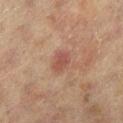Notes:
• biopsy status · catalogued during a skin exam; not biopsied
• subject · female, aged around 80
• acquisition · 15 mm crop, total-body photography
• site · the left lower leg
• lesion diameter · ~2.5 mm (longest diameter)